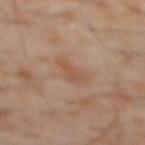workup=imaged on a skin check; not biopsied | image=total-body-photography crop, ~15 mm field of view | body site=the abdomen | patient=male, approximately 60 years of age | diameter=~3.5 mm (longest diameter).